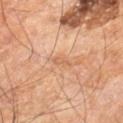| key | value |
|---|---|
| notes | catalogued during a skin exam; not biopsied |
| subject | male, aged 58–62 |
| body site | the right leg |
| image source | ~15 mm crop, total-body skin-cancer survey |